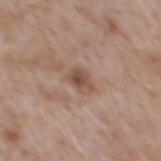Q: Was this lesion biopsied?
A: catalogued during a skin exam; not biopsied
Q: How was this image acquired?
A: ~15 mm tile from a whole-body skin photo
Q: What is the anatomic site?
A: the upper back
Q: What is the lesion's diameter?
A: ~3 mm (longest diameter)
Q: Patient demographics?
A: male, about 50 years old
Q: What lighting was used for the tile?
A: white-light illumination